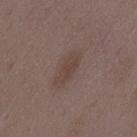The lesion was photographed on a routine skin check and not biopsied; there is no pathology result.
Longest diameter approximately 4.5 mm.
A female patient, aged around 35.
Automated image analysis of the tile measured an eccentricity of roughly 0.9 and a symmetry-axis asymmetry near 0.25. It also reported a mean CIELAB color near L≈42 a*≈15 b*≈21, about 7 CIELAB-L* units darker than the surrounding skin, and a normalized lesion–skin contrast near 6.5. It also reported a border-irregularity index near 3.5/10, a within-lesion color-variation index near 1/10, and radial color variation of about 0.5.
A region of skin cropped from a whole-body photographic capture, roughly 15 mm wide.
Imaged with white-light lighting.
The lesion is on the mid back.